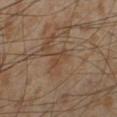Part of a total-body skin-imaging series; this lesion was reviewed on a skin check and was not flagged for biopsy. A male subject aged approximately 55. The lesion is on the left lower leg. The lesion's longest dimension is about 2.5 mm. Captured under cross-polarized illumination. A roughly 15 mm field-of-view crop from a total-body skin photograph.